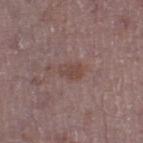Q: Is there a histopathology result?
A: imaged on a skin check; not biopsied
Q: Lesion location?
A: the left thigh
Q: How large is the lesion?
A: ~2.5 mm (longest diameter)
Q: Patient demographics?
A: male, aged 48 to 52
Q: What is the imaging modality?
A: total-body-photography crop, ~15 mm field of view